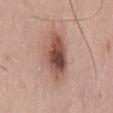follow-up: no biopsy performed (imaged during a skin exam); illumination: white-light; subject: male, about 50 years old; size: about 6 mm; image: total-body-photography crop, ~15 mm field of view; anatomic site: the lower back.